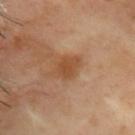Part of a total-body skin-imaging series; this lesion was reviewed on a skin check and was not flagged for biopsy. A female patient, about 60 years old. A 15 mm close-up tile from a total-body photography series done for melanoma screening. From the upper back.